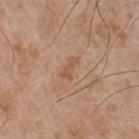Clinical impression:
The lesion was tiled from a total-body skin photograph and was not biopsied.
Context:
A 15 mm close-up extracted from a 3D total-body photography capture. The tile uses white-light illumination. A male subject roughly 65 years of age. The lesion is on the chest.